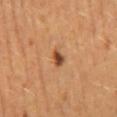{
  "site": "mid back",
  "image": {
    "source": "total-body photography crop",
    "field_of_view_mm": 15
  },
  "lesion_size": {
    "long_diameter_mm_approx": 2.5
  },
  "patient": {
    "sex": "female"
  }
}